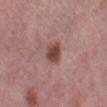Q: Was a biopsy performed?
A: catalogued during a skin exam; not biopsied
Q: Who is the patient?
A: female, approximately 50 years of age
Q: Lesion location?
A: the left lower leg
Q: What is the imaging modality?
A: ~15 mm tile from a whole-body skin photo